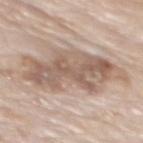– image-analysis metrics: an area of roughly 36 mm², an outline eccentricity of about 0.9 (0 = round, 1 = elongated), and a symmetry-axis asymmetry near 0.3; a mean CIELAB color near L≈59 a*≈15 b*≈26; a border-irregularity index near 5.5/10 and peripheral color asymmetry of about 2
– body site: the upper back
– lighting: white-light
– size: about 10.5 mm
– image source: ~15 mm crop, total-body skin-cancer survey
– subject: male, about 85 years old or older